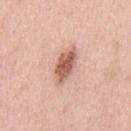Recorded during total-body skin imaging; not selected for excision or biopsy. The subject is a male roughly 40 years of age. The lesion is located on the mid back. A 15 mm crop from a total-body photograph taken for skin-cancer surveillance.